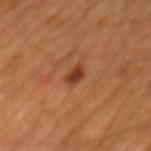workup: catalogued during a skin exam; not biopsied | acquisition: ~15 mm tile from a whole-body skin photo | patient: male, roughly 65 years of age | size: about 2.5 mm | tile lighting: cross-polarized illumination | anatomic site: the mid back | automated lesion analysis: about 10 CIELAB-L* units darker than the surrounding skin and a lesion-to-skin contrast of about 9 (normalized; higher = more distinct); an automated nevus-likeness rating near 90 out of 100 and a lesion-detection confidence of about 100/100.